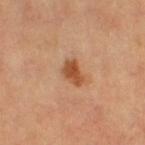<record>
<biopsy_status>not biopsied; imaged during a skin examination</biopsy_status>
<site>right thigh</site>
<lesion_size>
  <long_diameter_mm_approx>3.0</long_diameter_mm_approx>
</lesion_size>
<automated_metrics>
  <area_mm2_approx>5.0</area_mm2_approx>
  <shape_asymmetry>0.3</shape_asymmetry>
  <vs_skin_darker_L>12.0</vs_skin_darker_L>
  <vs_skin_contrast_norm>9.5</vs_skin_contrast_norm>
</automated_metrics>
<image>
  <source>total-body photography crop</source>
  <field_of_view_mm>15</field_of_view_mm>
</image>
<patient>
  <sex>female</sex>
  <age_approx>65</age_approx>
</patient>
</record>